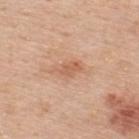Clinical impression: The lesion was tiled from a total-body skin photograph and was not biopsied. Context: Cropped from a whole-body photographic skin survey; the tile spans about 15 mm. The lesion-visualizer software estimated a mean CIELAB color near L≈61 a*≈23 b*≈33 and a lesion-to-skin contrast of about 6 (normalized; higher = more distinct). The software also gave border irregularity of about 4 on a 0–10 scale, internal color variation of about 1.5 on a 0–10 scale, and radial color variation of about 0.5. And it measured a classifier nevus-likeness of about 0/100. Imaged with white-light lighting. Located on the back. The patient is a male aged around 50. Approximately 3 mm at its widest.